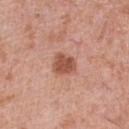This lesion was catalogued during total-body skin photography and was not selected for biopsy.
Captured under white-light illumination.
A 15 mm crop from a total-body photograph taken for skin-cancer surveillance.
Measured at roughly 3 mm in maximum diameter.
A male subject, approximately 80 years of age.
From the right lower leg.
An algorithmic analysis of the crop reported an area of roughly 6 mm² and a symmetry-axis asymmetry near 0.25. It also reported an average lesion color of about L≈53 a*≈25 b*≈30 (CIELAB) and a lesion-to-skin contrast of about 8.5 (normalized; higher = more distinct). The software also gave a border-irregularity index near 2/10 and a peripheral color-asymmetry measure near 0.5.